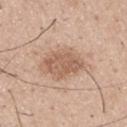{
  "image": {
    "source": "total-body photography crop",
    "field_of_view_mm": 15
  },
  "site": "upper back",
  "lesion_size": {
    "long_diameter_mm_approx": 7.0
  },
  "patient": {
    "sex": "male",
    "age_approx": 30
  }
}